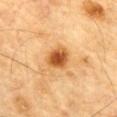Clinical summary:
On the chest. A male patient aged 83 or older. A close-up tile cropped from a whole-body skin photograph, about 15 mm across.